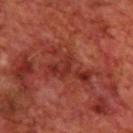biopsy status: imaged on a skin check; not biopsied